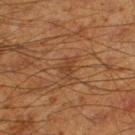The lesion was photographed on a routine skin check and not biopsied; there is no pathology result. A 15 mm crop from a total-body photograph taken for skin-cancer surveillance. From the left thigh. Measured at roughly 2.5 mm in maximum diameter. The patient is a male roughly 60 years of age. Captured under cross-polarized illumination.